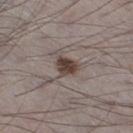This lesion was catalogued during total-body skin photography and was not selected for biopsy. Longest diameter approximately 3 mm. The patient is a male aged 68–72. The lesion is on the leg. This image is a 15 mm lesion crop taken from a total-body photograph.